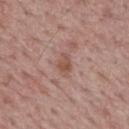Part of a total-body skin-imaging series; this lesion was reviewed on a skin check and was not flagged for biopsy.
Cropped from a total-body skin-imaging series; the visible field is about 15 mm.
Imaged with white-light lighting.
A male patient in their mid- to late 60s.
The lesion is on the mid back.
The lesion's longest dimension is about 2.5 mm.